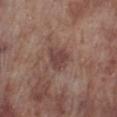{
  "site": "leg",
  "patient": {
    "sex": "male",
    "age_approx": 70
  },
  "lesion_size": {
    "long_diameter_mm_approx": 3.0
  },
  "automated_metrics": {
    "area_mm2_approx": 6.0,
    "eccentricity": 0.5,
    "shape_asymmetry": 0.25,
    "cielab_L": 42,
    "cielab_a": 20,
    "cielab_b": 21
  },
  "image": {
    "source": "total-body photography crop",
    "field_of_view_mm": 15
  }
}